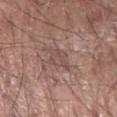notes: imaged on a skin check; not biopsied | subject: male, aged 68 to 72 | acquisition: 15 mm crop, total-body photography | lesion size: ~3 mm (longest diameter) | anatomic site: the right forearm | lighting: white-light illumination.